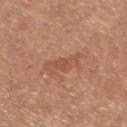Clinical summary:
This image is a 15 mm lesion crop taken from a total-body photograph. The recorded lesion diameter is about 4.5 mm. A female subject, approximately 65 years of age. The lesion is located on the left lower leg. The tile uses white-light illumination. Automated tile analysis of the lesion measured an area of roughly 6 mm² and a shape eccentricity near 0.95. And it measured a nevus-likeness score of about 0/100 and a detector confidence of about 100 out of 100 that the crop contains a lesion.